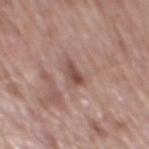The lesion was tiled from a total-body skin photograph and was not biopsied. The lesion is on the mid back. A male subject, about 70 years old. Longest diameter approximately 2.5 mm. A roughly 15 mm field-of-view crop from a total-body skin photograph. This is a white-light tile.